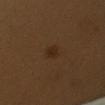Q: Was this lesion biopsied?
A: catalogued during a skin exam; not biopsied
Q: How large is the lesion?
A: about 2.5 mm
Q: What did automated image analysis measure?
A: a lesion–skin lightness drop of about 6 and a normalized lesion–skin contrast near 6.5
Q: What kind of image is this?
A: 15 mm crop, total-body photography
Q: Where on the body is the lesion?
A: the left upper arm
Q: Illumination type?
A: cross-polarized illumination
Q: Who is the patient?
A: female, aged 38 to 42